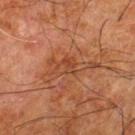<case>
  <biopsy_status>not biopsied; imaged during a skin examination</biopsy_status>
  <lighting>cross-polarized</lighting>
  <site>left thigh</site>
  <lesion_size>
    <long_diameter_mm_approx>6.5</long_diameter_mm_approx>
  </lesion_size>
  <image>
    <source>total-body photography crop</source>
    <field_of_view_mm>15</field_of_view_mm>
  </image>
  <patient>
    <sex>male</sex>
    <age_approx>80</age_approx>
  </patient>
</case>